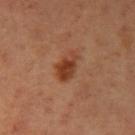The lesion was tiled from a total-body skin photograph and was not biopsied. A male subject, in their mid-30s. From the arm. The recorded lesion diameter is about 3.5 mm. A 15 mm close-up tile from a total-body photography series done for melanoma screening. Imaged with cross-polarized lighting.